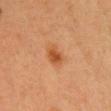{
  "biopsy_status": "not biopsied; imaged during a skin examination",
  "patient": {
    "sex": "female",
    "age_approx": 40
  },
  "lighting": "cross-polarized",
  "image": {
    "source": "total-body photography crop",
    "field_of_view_mm": 15
  },
  "site": "head or neck"
}